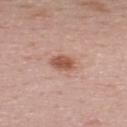Case summary:
– biopsy status: no biopsy performed (imaged during a skin exam)
– image source: ~15 mm tile from a whole-body skin photo
– automated lesion analysis: an area of roughly 5.5 mm², an outline eccentricity of about 0.65 (0 = round, 1 = elongated), and a shape-asymmetry score of about 0.25 (0 = symmetric); a border-irregularity index near 2/10
– anatomic site: the back
– patient: male, aged 23 to 27
– tile lighting: white-light